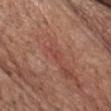<case>
  <biopsy_status>not biopsied; imaged during a skin examination</biopsy_status>
  <site>chest</site>
  <image>
    <source>total-body photography crop</source>
    <field_of_view_mm>15</field_of_view_mm>
  </image>
  <patient>
    <sex>male</sex>
    <age_approx>70</age_approx>
  </patient>
</case>